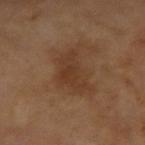patient: male, roughly 65 years of age
tile lighting: cross-polarized
anatomic site: the left arm
automated metrics: an area of roughly 11 mm², an outline eccentricity of about 0.8 (0 = round, 1 = elongated), and a shape-asymmetry score of about 0.4 (0 = symmetric); an average lesion color of about L≈34 a*≈18 b*≈29 (CIELAB), about 6 CIELAB-L* units darker than the surrounding skin, and a normalized lesion–skin contrast near 6; a border-irregularity rating of about 5/10 and radial color variation of about 1; a classifier nevus-likeness of about 5/100 and lesion-presence confidence of about 100/100
image: ~15 mm crop, total-body skin-cancer survey